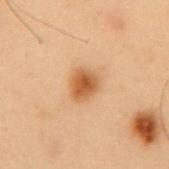{
  "image": {
    "source": "total-body photography crop",
    "field_of_view_mm": 15
  },
  "patient": {
    "sex": "male",
    "age_approx": 55
  },
  "site": "back"
}